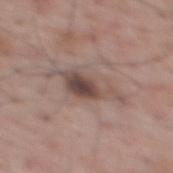An algorithmic analysis of the crop reported an area of roughly 9 mm² and a shape eccentricity near 0.9. The software also gave a border-irregularity index near 7/10 and a color-variation rating of about 5.5/10. A male patient, aged 68 to 72. Cropped from a total-body skin-imaging series; the visible field is about 15 mm. The tile uses white-light illumination. The lesion is on the upper back.